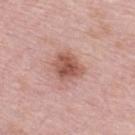The lesion is on the upper back. A female patient, in their mid-60s. Cropped from a whole-body photographic skin survey; the tile spans about 15 mm.